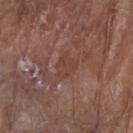Q: Was this lesion biopsied?
A: catalogued during a skin exam; not biopsied
Q: Lesion size?
A: ≈3 mm
Q: What is the anatomic site?
A: the right forearm
Q: How was this image acquired?
A: total-body-photography crop, ~15 mm field of view
Q: What did automated image analysis measure?
A: a lesion area of about 5.5 mm² and a shape-asymmetry score of about 0.35 (0 = symmetric); a within-lesion color-variation index near 2/10 and radial color variation of about 0.5; an automated nevus-likeness rating near 0 out of 100 and lesion-presence confidence of about 60/100
Q: Who is the patient?
A: male, in their mid- to late 80s or older